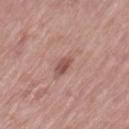body site: the left thigh
automated lesion analysis: an average lesion color of about L≈56 a*≈21 b*≈24 (CIELAB), a lesion–skin lightness drop of about 8, and a lesion-to-skin contrast of about 5.5 (normalized; higher = more distinct); a nevus-likeness score of about 5/100 and lesion-presence confidence of about 100/100
diameter: ~4.5 mm (longest diameter)
lighting: white-light
image: 15 mm crop, total-body photography
patient: female, aged around 65